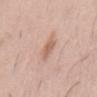follow-up: catalogued during a skin exam; not biopsied | location: the abdomen | patient: male, approximately 50 years of age | acquisition: ~15 mm crop, total-body skin-cancer survey | illumination: white-light | lesion size: about 2.5 mm | TBP lesion metrics: a mean CIELAB color near L≈62 a*≈21 b*≈29 and a normalized border contrast of about 6.